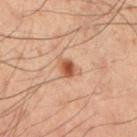Impression:
Captured during whole-body skin photography for melanoma surveillance; the lesion was not biopsied.
Image and clinical context:
Imaged with cross-polarized lighting. Automated tile analysis of the lesion measured a lesion area of about 4 mm² and a symmetry-axis asymmetry near 0.3. It also reported a lesion color around L≈42 a*≈20 b*≈27 in CIELAB and a normalized border contrast of about 8.5. A close-up tile cropped from a whole-body skin photograph, about 15 mm across. From the left lower leg. Approximately 2.5 mm at its widest. A male subject, aged 48–52.